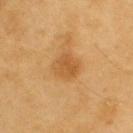Imaged during a routine full-body skin examination; the lesion was not biopsied and no histopathology is available. Automated image analysis of the tile measured an average lesion color of about L≈55 a*≈22 b*≈43 (CIELAB) and a normalized lesion–skin contrast near 6. The analysis additionally found a border-irregularity index near 1.5/10, a within-lesion color-variation index near 3/10, and peripheral color asymmetry of about 1. And it measured an automated nevus-likeness rating near 70 out of 100 and a detector confidence of about 100 out of 100 that the crop contains a lesion. From the upper back. A male subject aged 58–62. The lesion's longest dimension is about 3 mm. A lesion tile, about 15 mm wide, cut from a 3D total-body photograph.